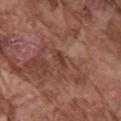Q: Is there a histopathology result?
A: catalogued during a skin exam; not biopsied
Q: Lesion location?
A: the right upper arm
Q: How large is the lesion?
A: ≈2.5 mm
Q: Patient demographics?
A: male, aged around 75
Q: Automated lesion metrics?
A: a lesion color around L≈39 a*≈22 b*≈26 in CIELAB, a lesion–skin lightness drop of about 8, and a lesion-to-skin contrast of about 7 (normalized; higher = more distinct); a border-irregularity index near 3/10, internal color variation of about 0 on a 0–10 scale, and radial color variation of about 0
Q: Illumination type?
A: white-light
Q: What is the imaging modality?
A: total-body-photography crop, ~15 mm field of view